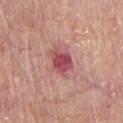Clinical impression: Recorded during total-body skin imaging; not selected for excision or biopsy. Context: Cropped from a whole-body photographic skin survey; the tile spans about 15 mm. The lesion is located on the chest. A male subject roughly 65 years of age. Automated image analysis of the tile measured an area of roughly 7.5 mm² and a shape eccentricity near 0.75. And it measured border irregularity of about 3 on a 0–10 scale and peripheral color asymmetry of about 1.5. Longest diameter approximately 4 mm.